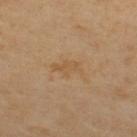The lesion was tiled from a total-body skin photograph and was not biopsied. On the upper back. A male patient aged around 55. This image is a 15 mm lesion crop taken from a total-body photograph. The lesion's longest dimension is about 4 mm. Captured under cross-polarized illumination. The total-body-photography lesion software estimated a classifier nevus-likeness of about 0/100 and lesion-presence confidence of about 100/100.